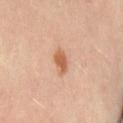Recorded during total-body skin imaging; not selected for excision or biopsy. The lesion is located on the lower back. The total-body-photography lesion software estimated an area of roughly 4 mm², a shape eccentricity near 0.75, and two-axis asymmetry of about 0.2. And it measured a lesion color around L≈60 a*≈23 b*≈36 in CIELAB and a normalized border contrast of about 8.5. It also reported a border-irregularity rating of about 2/10 and a peripheral color-asymmetry measure near 0.5. The analysis additionally found a nevus-likeness score of about 95/100 and lesion-presence confidence of about 100/100. The lesion's longest dimension is about 2.5 mm. This is a cross-polarized tile. A lesion tile, about 15 mm wide, cut from a 3D total-body photograph. The subject is a female aged approximately 40.